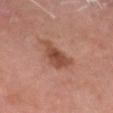About 4 mm across.
Located on the head or neck.
Automated tile analysis of the lesion measured a lesion area of about 9 mm², an outline eccentricity of about 0.6 (0 = round, 1 = elongated), and a symmetry-axis asymmetry near 0.4. And it measured an average lesion color of about L≈45 a*≈23 b*≈28 (CIELAB) and about 10 CIELAB-L* units darker than the surrounding skin. And it measured an automated nevus-likeness rating near 60 out of 100 and a detector confidence of about 100 out of 100 that the crop contains a lesion.
The subject is a male about 60 years old.
A 15 mm close-up extracted from a 3D total-body photography capture.